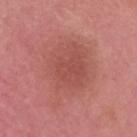Part of a total-body skin-imaging series; this lesion was reviewed on a skin check and was not flagged for biopsy. About 8 mm across. A close-up tile cropped from a whole-body skin photograph, about 15 mm across. A male patient aged 28–32. Located on the head or neck. Imaged with white-light lighting.